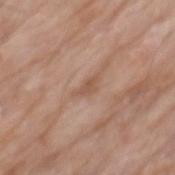notes: total-body-photography surveillance lesion; no biopsy | lighting: white-light | automated lesion analysis: a footprint of about 2.5 mm², an outline eccentricity of about 0.9 (0 = round, 1 = elongated), and a symmetry-axis asymmetry near 0.4; a border-irregularity rating of about 4.5/10 and a color-variation rating of about 0/10; an automated nevus-likeness rating near 0 out of 100 and a lesion-detection confidence of about 100/100 | image: ~15 mm tile from a whole-body skin photo | lesion size: ≈2.5 mm | location: the back | subject: male, about 80 years old.